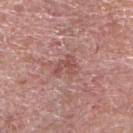Assessment:
Part of a total-body skin-imaging series; this lesion was reviewed on a skin check and was not flagged for biopsy.
Background:
From the right upper arm. A lesion tile, about 15 mm wide, cut from a 3D total-body photograph. A female subject roughly 60 years of age.